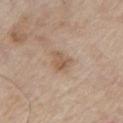Imaged during a routine full-body skin examination; the lesion was not biopsied and no histopathology is available.
The lesion is on the chest.
Cropped from a total-body skin-imaging series; the visible field is about 15 mm.
The tile uses white-light illumination.
A male subject, aged 78 to 82.